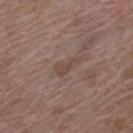This lesion was catalogued during total-body skin photography and was not selected for biopsy. Imaged with white-light lighting. A female patient aged 68–72. The total-body-photography lesion software estimated a lesion color around L≈45 a*≈15 b*≈24 in CIELAB, a lesion–skin lightness drop of about 6, and a normalized lesion–skin contrast near 6. A 15 mm crop from a total-body photograph taken for skin-cancer surveillance. Measured at roughly 3 mm in maximum diameter. From the left thigh.